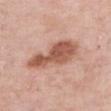Impression: Part of a total-body skin-imaging series; this lesion was reviewed on a skin check and was not flagged for biopsy. Image and clinical context: Located on the chest. The lesion-visualizer software estimated an area of roughly 15 mm², a shape eccentricity near 0.9, and two-axis asymmetry of about 0.35. The software also gave a border-irregularity rating of about 4/10 and a peripheral color-asymmetry measure near 1.5. The software also gave an automated nevus-likeness rating near 15 out of 100 and a lesion-detection confidence of about 100/100. A female subject in their mid- to late 60s. Imaged with white-light lighting. A roughly 15 mm field-of-view crop from a total-body skin photograph.